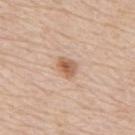| feature | finding |
|---|---|
| biopsy status | imaged on a skin check; not biopsied |
| image source | ~15 mm crop, total-body skin-cancer survey |
| TBP lesion metrics | two-axis asymmetry of about 0.2; a mean CIELAB color near L≈60 a*≈20 b*≈33, a lesion–skin lightness drop of about 12, and a lesion-to-skin contrast of about 8 (normalized; higher = more distinct) |
| body site | the back |
| size | ≈2.5 mm |
| subject | male, about 80 years old |
| lighting | white-light |